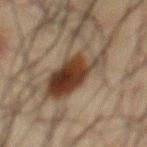Q: Is there a histopathology result?
A: imaged on a skin check; not biopsied
Q: What is the imaging modality?
A: 15 mm crop, total-body photography
Q: How large is the lesion?
A: about 8 mm
Q: What lighting was used for the tile?
A: cross-polarized
Q: What are the patient's age and sex?
A: male, about 60 years old
Q: What is the anatomic site?
A: the chest